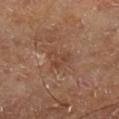{"biopsy_status": "not biopsied; imaged during a skin examination", "patient": {"sex": "male", "age_approx": 70}, "image": {"source": "total-body photography crop", "field_of_view_mm": 15}, "site": "left lower leg"}